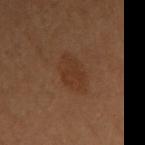biopsy status — total-body-photography surveillance lesion; no biopsy
site — the upper back
imaging modality — total-body-photography crop, ~15 mm field of view
image-analysis metrics — a footprint of about 10 mm², an eccentricity of roughly 0.85, and two-axis asymmetry of about 0.25; roughly 5 lightness units darker than nearby skin and a normalized border contrast of about 5.5; an automated nevus-likeness rating near 65 out of 100
subject — female, aged around 50
illumination — cross-polarized illumination